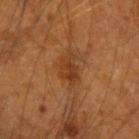Clinical impression: No biopsy was performed on this lesion — it was imaged during a full skin examination and was not determined to be concerning. Clinical summary: The lesion is on the arm. Cropped from a whole-body photographic skin survey; the tile spans about 15 mm. A male patient, aged 53 to 57.